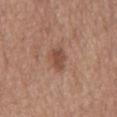Notes:
– lighting: white-light
– acquisition: total-body-photography crop, ~15 mm field of view
– anatomic site: the back
– patient: female, aged approximately 65
– automated metrics: a footprint of about 5 mm², an outline eccentricity of about 0.7 (0 = round, 1 = elongated), and a shape-asymmetry score of about 0.2 (0 = symmetric); an average lesion color of about L≈48 a*≈21 b*≈28 (CIELAB), about 9 CIELAB-L* units darker than the surrounding skin, and a normalized lesion–skin contrast near 7